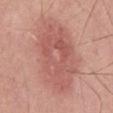No biopsy was performed on this lesion — it was imaged during a full skin examination and was not determined to be concerning. Imaged with white-light lighting. Automated image analysis of the tile measured a lesion color around L≈57 a*≈26 b*≈26 in CIELAB, a lesion–skin lightness drop of about 9, and a normalized lesion–skin contrast near 6. The software also gave a border-irregularity index near 2/10, internal color variation of about 4 on a 0–10 scale, and radial color variation of about 1.5. It also reported a nevus-likeness score of about 60/100 and a lesion-detection confidence of about 100/100. A male patient in their mid-50s. Longest diameter approximately 9.5 mm. The lesion is on the abdomen. Cropped from a total-body skin-imaging series; the visible field is about 15 mm.